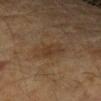Clinical impression: This lesion was catalogued during total-body skin photography and was not selected for biopsy. Acquisition and patient details: A female patient aged around 60. This is a cross-polarized tile. On the right forearm. Cropped from a whole-body photographic skin survey; the tile spans about 15 mm. The lesion's longest dimension is about 3.5 mm.